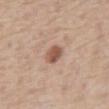The recorded lesion diameter is about 2.5 mm.
A 15 mm close-up tile from a total-body photography series done for melanoma screening.
The lesion is located on the abdomen.
A male subject, in their 70s.
The total-body-photography lesion software estimated an eccentricity of roughly 0.6 and a shape-asymmetry score of about 0.15 (0 = symmetric). And it measured an average lesion color of about L≈54 a*≈21 b*≈28 (CIELAB), roughly 13 lightness units darker than nearby skin, and a normalized border contrast of about 8.5. The analysis additionally found a border-irregularity rating of about 1/10, internal color variation of about 3 on a 0–10 scale, and peripheral color asymmetry of about 1. It also reported lesion-presence confidence of about 100/100.
This is a white-light tile.